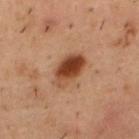Impression: No biopsy was performed on this lesion — it was imaged during a full skin examination and was not determined to be concerning. Image and clinical context: The subject is a male in their mid-50s. A roughly 15 mm field-of-view crop from a total-body skin photograph. Measured at roughly 4.5 mm in maximum diameter. Captured under cross-polarized illumination. On the upper back.